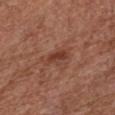biopsy status: total-body-photography surveillance lesion; no biopsy | imaging modality: 15 mm crop, total-body photography | lesion diameter: about 3 mm | anatomic site: the chest | patient: female, about 75 years old.